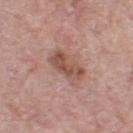biopsy status = imaged on a skin check; not biopsied
patient = female, in their mid-60s
imaging modality = 15 mm crop, total-body photography
location = the left thigh